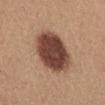– body site — the abdomen
– image source — total-body-photography crop, ~15 mm field of view
– subject — female, aged 53–57